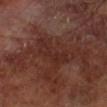Clinical summary:
An algorithmic analysis of the crop reported an area of roughly 10 mm², a shape eccentricity near 0.9, and a shape-asymmetry score of about 0.5 (0 = symmetric). It also reported a nevus-likeness score of about 0/100 and a detector confidence of about 95 out of 100 that the crop contains a lesion. A male patient, aged 68–72. This is a cross-polarized tile. A roughly 15 mm field-of-view crop from a total-body skin photograph. Located on the right lower leg.